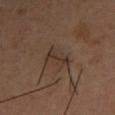  biopsy_status: not biopsied; imaged during a skin examination
  automated_metrics:
    area_mm2_approx: 4.5
    eccentricity: 0.95
    shape_asymmetry: 0.4
    vs_skin_contrast_norm: 6.5
    lesion_detection_confidence_0_100: 65
  patient:
    sex: male
    age_approx: 40
  lighting: cross-polarized
  image:
    source: total-body photography crop
    field_of_view_mm: 15
  site: right upper arm